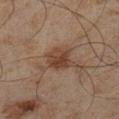The lesion was photographed on a routine skin check and not biopsied; there is no pathology result. The subject is a male aged approximately 45. Cropped from a total-body skin-imaging series; the visible field is about 15 mm. The lesion is located on the left lower leg.